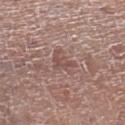The lesion was photographed on a routine skin check and not biopsied; there is no pathology result. The subject is a male approximately 80 years of age. Automated tile analysis of the lesion measured a mean CIELAB color near L≈50 a*≈19 b*≈22, roughly 8 lightness units darker than nearby skin, and a normalized lesion–skin contrast near 5.5. And it measured internal color variation of about 1.5 on a 0–10 scale and a peripheral color-asymmetry measure near 0.5. It also reported lesion-presence confidence of about 95/100. This is a white-light tile. The recorded lesion diameter is about 3 mm. Cropped from a whole-body photographic skin survey; the tile spans about 15 mm. From the left lower leg.